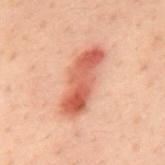Clinical impression: Captured during whole-body skin photography for melanoma surveillance; the lesion was not biopsied. Context: This image is a 15 mm lesion crop taken from a total-body photograph. The lesion is on the mid back. A male patient, aged 38–42. Imaged with cross-polarized lighting. About 7.5 mm across.